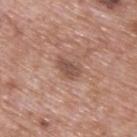Assessment: The lesion was tiled from a total-body skin photograph and was not biopsied. Background: The patient is a male aged 68–72. A close-up tile cropped from a whole-body skin photograph, about 15 mm across. The lesion is located on the upper back. Captured under white-light illumination. Measured at roughly 3 mm in maximum diameter.